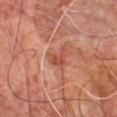No biopsy was performed on this lesion — it was imaged during a full skin examination and was not determined to be concerning. A lesion tile, about 15 mm wide, cut from a 3D total-body photograph. The tile uses cross-polarized illumination. A male subject roughly 60 years of age. From the front of the torso.